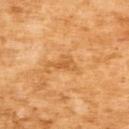This lesion was catalogued during total-body skin photography and was not selected for biopsy.
Measured at roughly 3.5 mm in maximum diameter.
The tile uses cross-polarized illumination.
A male patient aged 63–67.
A close-up tile cropped from a whole-body skin photograph, about 15 mm across.